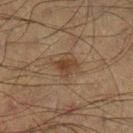Part of a total-body skin-imaging series; this lesion was reviewed on a skin check and was not flagged for biopsy.
Captured under cross-polarized illumination.
About 3 mm across.
A 15 mm close-up tile from a total-body photography series done for melanoma screening.
The lesion is on the left lower leg.
The subject is a male aged approximately 50.